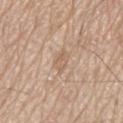biopsy status: no biopsy performed (imaged during a skin exam)
subject: male, approximately 80 years of age
acquisition: 15 mm crop, total-body photography
illumination: white-light
lesion diameter: ≈2.5 mm
anatomic site: the mid back